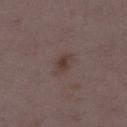This lesion was catalogued during total-body skin photography and was not selected for biopsy. A 15 mm close-up tile from a total-body photography series done for melanoma screening. On the right thigh. This is a white-light tile. The recorded lesion diameter is about 3.5 mm. A female subject, in their mid-30s.